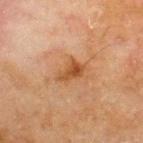Assessment:
Part of a total-body skin-imaging series; this lesion was reviewed on a skin check and was not flagged for biopsy.